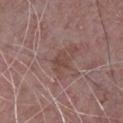Assessment: Captured during whole-body skin photography for melanoma surveillance; the lesion was not biopsied. Acquisition and patient details: A region of skin cropped from a whole-body photographic capture, roughly 15 mm wide. Approximately 2.5 mm at its widest. From the chest. Imaged with white-light lighting. A male subject, aged approximately 65.